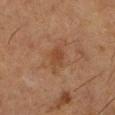biopsy_status: not biopsied; imaged during a skin examination
patient:
  sex: female
  age_approx: 50
image:
  source: total-body photography crop
  field_of_view_mm: 15
site: left lower leg
lesion_size:
  long_diameter_mm_approx: 2.5